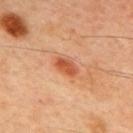Assessment: No biopsy was performed on this lesion — it was imaged during a full skin examination and was not determined to be concerning. Acquisition and patient details: On the upper back. A lesion tile, about 15 mm wide, cut from a 3D total-body photograph. A male patient aged 43 to 47. Measured at roughly 3 mm in maximum diameter. Imaged with cross-polarized lighting. An algorithmic analysis of the crop reported an area of roughly 4 mm², an eccentricity of roughly 0.8, and a shape-asymmetry score of about 0.2 (0 = symmetric). It also reported a lesion color around L≈52 a*≈30 b*≈38 in CIELAB, a lesion–skin lightness drop of about 12, and a normalized lesion–skin contrast near 8.5. And it measured internal color variation of about 3 on a 0–10 scale and peripheral color asymmetry of about 1.